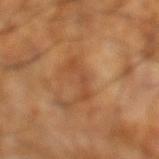Recorded during total-body skin imaging; not selected for excision or biopsy. A roughly 15 mm field-of-view crop from a total-body skin photograph. The subject is a male aged approximately 60. Located on the left lower leg.